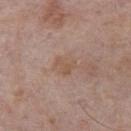• follow-up · no biopsy performed (imaged during a skin exam)
• site · the chest
• imaging modality · ~15 mm tile from a whole-body skin photo
• subject · male, aged 73–77
• lighting · white-light illumination
• automated metrics · a lesion area of about 5 mm² and two-axis asymmetry of about 0.3; an average lesion color of about L≈54 a*≈17 b*≈28 (CIELAB) and about 6 CIELAB-L* units darker than the surrounding skin
• lesion size · ≈2.5 mm